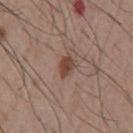  biopsy_status: not biopsied; imaged during a skin examination
  image:
    source: total-body photography crop
    field_of_view_mm: 15
  site: chest
  lesion_size:
    long_diameter_mm_approx: 2.5
  patient:
    sex: male
    age_approx: 55
  lighting: white-light